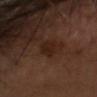Q: What is the anatomic site?
A: the head or neck
Q: What is the imaging modality?
A: 15 mm crop, total-body photography
Q: What is the lesion's diameter?
A: about 2.5 mm
Q: Patient demographics?
A: male, aged 63–67